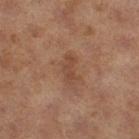Q: Was a biopsy performed?
A: catalogued during a skin exam; not biopsied
Q: Lesion size?
A: about 3.5 mm
Q: What lighting was used for the tile?
A: cross-polarized illumination
Q: What are the patient's age and sex?
A: female, approximately 60 years of age
Q: What did automated image analysis measure?
A: an eccentricity of roughly 0.9 and a shape-asymmetry score of about 0.35 (0 = symmetric); a color-variation rating of about 1.5/10 and peripheral color asymmetry of about 0.5; a detector confidence of about 100 out of 100 that the crop contains a lesion
Q: Lesion location?
A: the left thigh
Q: What is the imaging modality?
A: ~15 mm tile from a whole-body skin photo